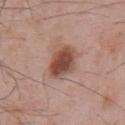Notes:
- workup — imaged on a skin check; not biopsied
- automated metrics — a footprint of about 11 mm², an outline eccentricity of about 0.7 (0 = round, 1 = elongated), and two-axis asymmetry of about 0.15; a nevus-likeness score of about 100/100
- anatomic site — the chest
- imaging modality — ~15 mm tile from a whole-body skin photo
- patient — male, in their mid-60s